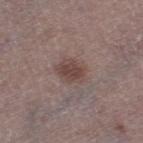Q: Is there a histopathology result?
A: catalogued during a skin exam; not biopsied
Q: What lighting was used for the tile?
A: white-light illumination
Q: How large is the lesion?
A: about 3 mm
Q: What are the patient's age and sex?
A: male, aged 73–77
Q: Where on the body is the lesion?
A: the right thigh
Q: What kind of image is this?
A: ~15 mm tile from a whole-body skin photo